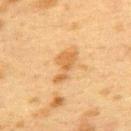biopsy status: catalogued during a skin exam; not biopsied | site: the back | diameter: ≈4 mm | tile lighting: cross-polarized | TBP lesion metrics: an average lesion color of about L≈53 a*≈19 b*≈39 (CIELAB), roughly 9 lightness units darker than nearby skin, and a lesion-to-skin contrast of about 7 (normalized; higher = more distinct); an automated nevus-likeness rating near 15 out of 100 and lesion-presence confidence of about 100/100 | subject: female, aged around 40 | image source: total-body-photography crop, ~15 mm field of view.